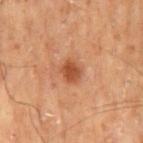No biopsy was performed on this lesion — it was imaged during a full skin examination and was not determined to be concerning. Cropped from a total-body skin-imaging series; the visible field is about 15 mm. The lesion is located on the mid back. This is a cross-polarized tile. Longest diameter approximately 2.5 mm. The lesion-visualizer software estimated an outline eccentricity of about 0.55 (0 = round, 1 = elongated) and a shape-asymmetry score of about 0.2 (0 = symmetric). And it measured a mean CIELAB color near L≈38 a*≈21 b*≈29, about 9 CIELAB-L* units darker than the surrounding skin, and a lesion-to-skin contrast of about 8 (normalized; higher = more distinct). It also reported a border-irregularity index near 2/10, a within-lesion color-variation index near 2/10, and peripheral color asymmetry of about 0.5. A male patient approximately 70 years of age.